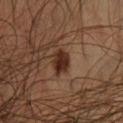Assessment:
The lesion was photographed on a routine skin check and not biopsied; there is no pathology result.
Context:
A male patient, in their mid-30s. A 15 mm close-up tile from a total-body photography series done for melanoma screening. The lesion is located on the front of the torso.